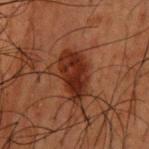Part of a total-body skin-imaging series; this lesion was reviewed on a skin check and was not flagged for biopsy.
Cropped from a total-body skin-imaging series; the visible field is about 15 mm.
An algorithmic analysis of the crop reported an eccentricity of roughly 0.9 and two-axis asymmetry of about 0.2. The analysis additionally found roughly 10 lightness units darker than nearby skin and a normalized lesion–skin contrast near 11. The analysis additionally found a nevus-likeness score of about 95/100 and lesion-presence confidence of about 100/100.
A male patient, in their 50s.
On the back.
The lesion's longest dimension is about 6 mm.